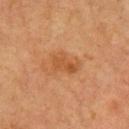{
  "biopsy_status": "not biopsied; imaged during a skin examination",
  "site": "upper back",
  "lighting": "cross-polarized",
  "image": {
    "source": "total-body photography crop",
    "field_of_view_mm": 15
  },
  "patient": {
    "sex": "male",
    "age_approx": 60
  },
  "lesion_size": {
    "long_diameter_mm_approx": 3.5
  }
}